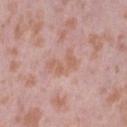follow-up — no biopsy performed (imaged during a skin exam); image source — ~15 mm crop, total-body skin-cancer survey; subject — female, roughly 40 years of age; tile lighting — white-light illumination; body site — the right thigh.